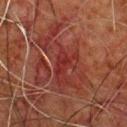Impression: The lesion was tiled from a total-body skin photograph and was not biopsied. Background: The lesion is located on the chest. Cropped from a total-body skin-imaging series; the visible field is about 15 mm. Imaged with cross-polarized lighting. The subject is a male aged around 80. Approximately 9.5 mm at its widest. An algorithmic analysis of the crop reported an average lesion color of about L≈28 a*≈24 b*≈22 (CIELAB), roughly 6 lightness units darker than nearby skin, and a normalized border contrast of about 6.5. The analysis additionally found a classifier nevus-likeness of about 0/100 and a detector confidence of about 65 out of 100 that the crop contains a lesion.